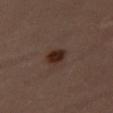No biopsy was performed on this lesion — it was imaged during a full skin examination and was not determined to be concerning. The lesion's longest dimension is about 2.5 mm. Imaged with cross-polarized lighting. On the right thigh. The subject is a female in their mid- to late 30s. The total-body-photography lesion software estimated border irregularity of about 1.5 on a 0–10 scale and radial color variation of about 0.5. A 15 mm crop from a total-body photograph taken for skin-cancer surveillance.